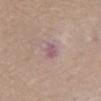Captured during whole-body skin photography for melanoma surveillance; the lesion was not biopsied. A female patient aged around 70. The recorded lesion diameter is about 2.5 mm. The tile uses white-light illumination. A lesion tile, about 15 mm wide, cut from a 3D total-body photograph. The lesion is located on the abdomen.